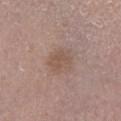Impression: This lesion was catalogued during total-body skin photography and was not selected for biopsy. Image and clinical context: The lesion is on the left lower leg. A male subject, about 55 years old. The tile uses white-light illumination. The total-body-photography lesion software estimated a within-lesion color-variation index near 2/10. It also reported an automated nevus-likeness rating near 5 out of 100 and a detector confidence of about 100 out of 100 that the crop contains a lesion. Approximately 3.5 mm at its widest. A 15 mm close-up tile from a total-body photography series done for melanoma screening.